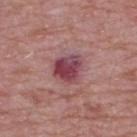notes = imaged on a skin check; not biopsied | subject = male, in their mid-60s | acquisition = ~15 mm crop, total-body skin-cancer survey | body site = the upper back.